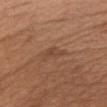Captured during whole-body skin photography for melanoma surveillance; the lesion was not biopsied. Longest diameter approximately 3.5 mm. The lesion is on the chest. Captured under white-light illumination. A female subject, in their mid-50s. An algorithmic analysis of the crop reported about 6 CIELAB-L* units darker than the surrounding skin and a lesion-to-skin contrast of about 5 (normalized; higher = more distinct). It also reported a classifier nevus-likeness of about 0/100 and a lesion-detection confidence of about 95/100. Cropped from a total-body skin-imaging series; the visible field is about 15 mm.